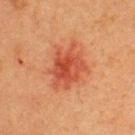workup — catalogued during a skin exam; not biopsied | lesion size — about 5.5 mm | patient — male, approximately 40 years of age | automated lesion analysis — a shape eccentricity near 0.6 and a symmetry-axis asymmetry near 0.3; a within-lesion color-variation index near 5/10 and a peripheral color-asymmetry measure near 1.5 | anatomic site — the upper back | illumination — cross-polarized illumination | image — total-body-photography crop, ~15 mm field of view.